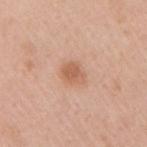biopsy status: catalogued during a skin exam; not biopsied | illumination: white-light | size: ~3 mm (longest diameter) | acquisition: total-body-photography crop, ~15 mm field of view | patient: male, approximately 60 years of age | site: the right upper arm.